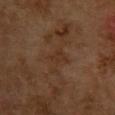Q: Was this lesion biopsied?
A: catalogued during a skin exam; not biopsied
Q: Who is the patient?
A: female, aged approximately 60
Q: How was the tile lit?
A: cross-polarized illumination
Q: What is the anatomic site?
A: the back
Q: What kind of image is this?
A: ~15 mm crop, total-body skin-cancer survey